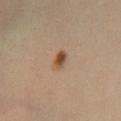Clinical impression: The lesion was tiled from a total-body skin photograph and was not biopsied. Acquisition and patient details: The lesion-visualizer software estimated an area of roughly 3.5 mm², an outline eccentricity of about 0.75 (0 = round, 1 = elongated), and a symmetry-axis asymmetry near 0.15. The analysis additionally found an average lesion color of about L≈48 a*≈18 b*≈32 (CIELAB), a lesion–skin lightness drop of about 11, and a normalized lesion–skin contrast near 9. It also reported a border-irregularity index near 1.5/10, a color-variation rating of about 4.5/10, and radial color variation of about 1.5. This is a cross-polarized tile. A 15 mm close-up extracted from a 3D total-body photography capture. The lesion is on the left lower leg. A female patient about 35 years old. Approximately 2.5 mm at its widest.